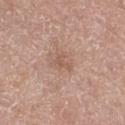This lesion was catalogued during total-body skin photography and was not selected for biopsy. Located on the leg. The patient is a female aged around 75. This image is a 15 mm lesion crop taken from a total-body photograph.